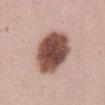Q: Was a biopsy performed?
A: imaged on a skin check; not biopsied
Q: How large is the lesion?
A: ≈6 mm
Q: Illumination type?
A: white-light illumination
Q: How was this image acquired?
A: total-body-photography crop, ~15 mm field of view
Q: Who is the patient?
A: female, aged 43 to 47
Q: What did automated image analysis measure?
A: a classifier nevus-likeness of about 60/100 and a detector confidence of about 100 out of 100 that the crop contains a lesion
Q: Lesion location?
A: the abdomen